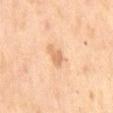Recorded during total-body skin imaging; not selected for excision or biopsy.
About 3.5 mm across.
Cropped from a whole-body photographic skin survey; the tile spans about 15 mm.
A female patient, in their 60s.
Imaged with cross-polarized lighting.
Located on the mid back.